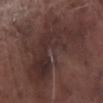Case summary:
- location · the left lower leg
- lighting · white-light
- image · total-body-photography crop, ~15 mm field of view
- subject · male, in their mid-70s
- lesion diameter · ~15 mm (longest diameter)